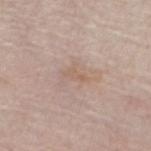Notes:
– workup — catalogued during a skin exam; not biopsied
– subject — female, in their mid-60s
– imaging modality — ~15 mm tile from a whole-body skin photo
– tile lighting — white-light illumination
– location — the left leg